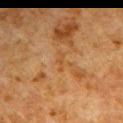notes: no biopsy performed (imaged during a skin exam) | body site: the right upper arm | image source: ~15 mm tile from a whole-body skin photo | diameter: ~3 mm (longest diameter) | subject: male, aged around 80 | tile lighting: cross-polarized illumination.